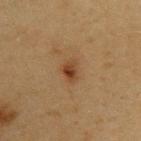<lesion>
<patient>
  <sex>female</sex>
  <age_approx>40</age_approx>
</patient>
<site>back</site>
<image>
  <source>total-body photography crop</source>
  <field_of_view_mm>15</field_of_view_mm>
</image>
<lighting>cross-polarized</lighting>
<lesion_size>
  <long_diameter_mm_approx>2.5</long_diameter_mm_approx>
</lesion_size>
<automated_metrics>
  <area_mm2_approx>3.5</area_mm2_approx>
  <eccentricity>0.7</eccentricity>
  <shape_asymmetry>0.35</shape_asymmetry>
  <cielab_L>35</cielab_L>
  <cielab_a>18</cielab_a>
  <cielab_b>30</cielab_b>
  <vs_skin_darker_L>9.0</vs_skin_darker_L>
  <nevus_likeness_0_100>90</nevus_likeness_0_100>
  <lesion_detection_confidence_0_100>100</lesion_detection_confidence_0_100>
</automated_metrics>
</lesion>